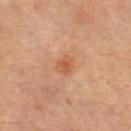The lesion was photographed on a routine skin check and not biopsied; there is no pathology result. The tile uses cross-polarized illumination. A female patient, in their mid- to late 50s. The lesion-visualizer software estimated a footprint of about 4 mm², a shape eccentricity near 0.7, and two-axis asymmetry of about 0.3. It also reported a nevus-likeness score of about 20/100 and a detector confidence of about 100 out of 100 that the crop contains a lesion. A 15 mm close-up extracted from a 3D total-body photography capture. The lesion is on the leg. Measured at roughly 2.5 mm in maximum diameter.